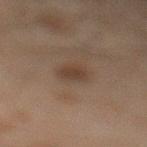biopsy status — no biopsy performed (imaged during a skin exam); image source — ~15 mm crop, total-body skin-cancer survey; body site — the leg; patient — male, roughly 45 years of age.